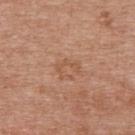The lesion was photographed on a routine skin check and not biopsied; there is no pathology result.
Longest diameter approximately 2.5 mm.
This is a white-light tile.
The total-body-photography lesion software estimated an average lesion color of about L≈54 a*≈22 b*≈33 (CIELAB), about 6 CIELAB-L* units darker than the surrounding skin, and a normalized border contrast of about 4.5. And it measured a border-irregularity rating of about 9/10, a within-lesion color-variation index near 0/10, and radial color variation of about 0. And it measured an automated nevus-likeness rating near 0 out of 100.
A region of skin cropped from a whole-body photographic capture, roughly 15 mm wide.
Located on the upper back.
The patient is a female in their 40s.